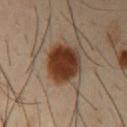workup — no biopsy performed (imaged during a skin exam)
automated lesion analysis — an area of roughly 16 mm² and a shape eccentricity near 0.5; a lesion color around L≈35 a*≈20 b*≈29 in CIELAB and a lesion-to-skin contrast of about 14 (normalized; higher = more distinct); a border-irregularity rating of about 1.5/10, a color-variation rating of about 4.5/10, and radial color variation of about 1.5; an automated nevus-likeness rating near 100 out of 100 and a detector confidence of about 100 out of 100 that the crop contains a lesion
subject — male, aged around 40
image — ~15 mm crop, total-body skin-cancer survey
body site — the right upper arm
lighting — cross-polarized
lesion diameter — ~5 mm (longest diameter)